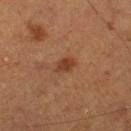Q: Was this lesion biopsied?
A: catalogued during a skin exam; not biopsied
Q: Who is the patient?
A: male, aged approximately 75
Q: Where on the body is the lesion?
A: the right thigh
Q: What is the lesion's diameter?
A: ≈2.5 mm
Q: How was this image acquired?
A: 15 mm crop, total-body photography
Q: What did automated image analysis measure?
A: an average lesion color of about L≈31 a*≈19 b*≈27 (CIELAB) and a normalized border contrast of about 7.5; a nevus-likeness score of about 90/100 and lesion-presence confidence of about 100/100